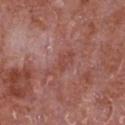Recorded during total-body skin imaging; not selected for excision or biopsy.
An algorithmic analysis of the crop reported a footprint of about 5 mm², a shape eccentricity near 0.9, and a shape-asymmetry score of about 0.45 (0 = symmetric). It also reported a within-lesion color-variation index near 1.5/10 and a peripheral color-asymmetry measure near 0.5. And it measured an automated nevus-likeness rating near 0 out of 100 and a lesion-detection confidence of about 100/100.
Cropped from a total-body skin-imaging series; the visible field is about 15 mm.
The lesion is located on the front of the torso.
A male subject, aged around 65.